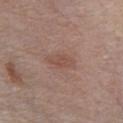workup=catalogued during a skin exam; not biopsied | image=~15 mm crop, total-body skin-cancer survey | site=the front of the torso | lesion diameter=about 4 mm | patient=male, approximately 80 years of age.